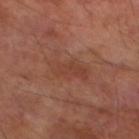Q: Was this lesion biopsied?
A: total-body-photography surveillance lesion; no biopsy
Q: What lighting was used for the tile?
A: cross-polarized illumination
Q: Patient demographics?
A: male, roughly 70 years of age
Q: Where on the body is the lesion?
A: the left lower leg
Q: How was this image acquired?
A: total-body-photography crop, ~15 mm field of view
Q: What is the lesion's diameter?
A: ≈5 mm